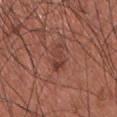Findings:
- biopsy status: catalogued during a skin exam; not biopsied
- anatomic site: the chest
- subject: male, aged 33 to 37
- image source: ~15 mm tile from a whole-body skin photo
- lighting: white-light illumination